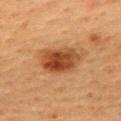{
  "biopsy_status": "not biopsied; imaged during a skin examination",
  "site": "upper back",
  "patient": {
    "sex": "female",
    "age_approx": 55
  },
  "lesion_size": {
    "long_diameter_mm_approx": 5.5
  },
  "image": {
    "source": "total-body photography crop",
    "field_of_view_mm": 15
  }
}